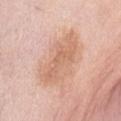Impression: Part of a total-body skin-imaging series; this lesion was reviewed on a skin check and was not flagged for biopsy. Clinical summary: The lesion-visualizer software estimated an average lesion color of about L≈66 a*≈21 b*≈32 (CIELAB), a lesion–skin lightness drop of about 9, and a lesion-to-skin contrast of about 6 (normalized; higher = more distinct). It also reported a border-irregularity rating of about 3.5/10 and a peripheral color-asymmetry measure near 1. The software also gave a classifier nevus-likeness of about 0/100 and lesion-presence confidence of about 100/100. About 7 mm across. The tile uses white-light illumination. The lesion is located on the abdomen. The patient is a female in their 50s. A region of skin cropped from a whole-body photographic capture, roughly 15 mm wide.